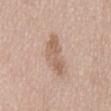| key | value |
|---|---|
| follow-up | catalogued during a skin exam; not biopsied |
| patient | male, aged approximately 75 |
| image source | ~15 mm tile from a whole-body skin photo |
| site | the back |
| illumination | white-light |